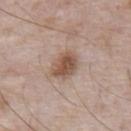Findings:
* follow-up — catalogued during a skin exam; not biopsied
* image-analysis metrics — an average lesion color of about L≈53 a*≈18 b*≈27 (CIELAB), a lesion–skin lightness drop of about 12, and a normalized border contrast of about 9; border irregularity of about 1.5 on a 0–10 scale, a within-lesion color-variation index near 4/10, and a peripheral color-asymmetry measure near 1; a classifier nevus-likeness of about 90/100 and a lesion-detection confidence of about 100/100
* imaging modality — ~15 mm crop, total-body skin-cancer survey
* body site — the abdomen
* subject — male, in their 70s
* diameter — ≈4 mm